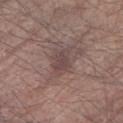<record>
<biopsy_status>not biopsied; imaged during a skin examination</biopsy_status>
<image>
  <source>total-body photography crop</source>
  <field_of_view_mm>15</field_of_view_mm>
</image>
<site>right forearm</site>
<patient>
  <sex>male</sex>
  <age_approx>80</age_approx>
</patient>
</record>